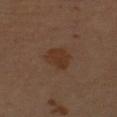Recorded during total-body skin imaging; not selected for excision or biopsy.
A roughly 15 mm field-of-view crop from a total-body skin photograph.
From the left upper arm.
The patient is a male aged 53–57.
Imaged with cross-polarized lighting.